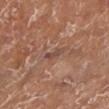  image:
    source: total-body photography crop
    field_of_view_mm: 15
  patient:
    sex: female
    age_approx: 75
  lighting: white-light
  site: left lower leg